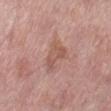Q: How was this image acquired?
A: 15 mm crop, total-body photography
Q: Lesion location?
A: the left lower leg
Q: What did automated image analysis measure?
A: a border-irregularity index near 4.5/10, internal color variation of about 3 on a 0–10 scale, and peripheral color asymmetry of about 1
Q: What lighting was used for the tile?
A: white-light illumination
Q: Lesion size?
A: about 3.5 mm
Q: What are the patient's age and sex?
A: female, roughly 60 years of age